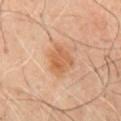Clinical impression: Recorded during total-body skin imaging; not selected for excision or biopsy. Clinical summary: Cropped from a total-body skin-imaging series; the visible field is about 15 mm. The lesion is located on the chest. The subject is approximately 65 years of age. The lesion's longest dimension is about 4 mm.